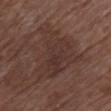  biopsy_status: not biopsied; imaged during a skin examination
  lesion_size:
    long_diameter_mm_approx: 8.0
  automated_metrics:
    area_mm2_approx: 26.0
    eccentricity: 0.65
    shape_asymmetry: 0.5
    vs_skin_darker_L: 6.0
    vs_skin_contrast_norm: 6.0
    border_irregularity_0_10: 7.0
    color_variation_0_10: 3.5
    peripheral_color_asymmetry: 1.0
    nevus_likeness_0_100: 0
    lesion_detection_confidence_0_100: 100
  lighting: white-light
  patient:
    sex: female
    age_approx: 80
  image:
    source: total-body photography crop
    field_of_view_mm: 15
  site: chest